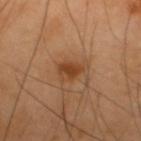biopsy_status: not biopsied; imaged during a skin examination
site: upper back
lighting: cross-polarized
lesion_size:
  long_diameter_mm_approx: 2.5
image:
  source: total-body photography crop
  field_of_view_mm: 15
patient:
  sex: male
  age_approx: 40